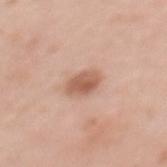Impression: This lesion was catalogued during total-body skin photography and was not selected for biopsy. Image and clinical context: The patient is a female roughly 50 years of age. Located on the back. Cropped from a whole-body photographic skin survey; the tile spans about 15 mm.